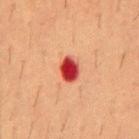workup — total-body-photography surveillance lesion; no biopsy
acquisition — ~15 mm crop, total-body skin-cancer survey
anatomic site — the mid back
patient — male, approximately 55 years of age
diameter — ≈3 mm